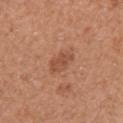The lesion was tiled from a total-body skin photograph and was not biopsied. The lesion is on the right upper arm. Measured at roughly 3.5 mm in maximum diameter. A female subject about 50 years old. Captured under white-light illumination. This image is a 15 mm lesion crop taken from a total-body photograph.